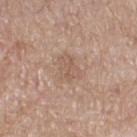This lesion was catalogued during total-body skin photography and was not selected for biopsy.
A female patient in their mid- to late 50s.
Located on the right thigh.
The lesion-visualizer software estimated an area of roughly 6 mm², an eccentricity of roughly 0.8, and a symmetry-axis asymmetry near 0.3. The analysis additionally found a border-irregularity index near 3.5/10 and internal color variation of about 2.5 on a 0–10 scale. The analysis additionally found a nevus-likeness score of about 0/100 and lesion-presence confidence of about 100/100.
A roughly 15 mm field-of-view crop from a total-body skin photograph.
Longest diameter approximately 3.5 mm.
The tile uses white-light illumination.